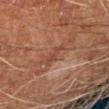Q: Is there a histopathology result?
A: imaged on a skin check; not biopsied
Q: Illumination type?
A: cross-polarized illumination
Q: What is the imaging modality?
A: 15 mm crop, total-body photography
Q: What are the patient's age and sex?
A: male, aged 58 to 62
Q: What is the lesion's diameter?
A: ≈3 mm
Q: Where on the body is the lesion?
A: the left arm